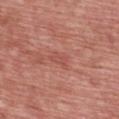The lesion was tiled from a total-body skin photograph and was not biopsied. Imaged with white-light lighting. From the upper back. A roughly 15 mm field-of-view crop from a total-body skin photograph. An algorithmic analysis of the crop reported a lesion–skin lightness drop of about 6 and a normalized border contrast of about 5. And it measured border irregularity of about 4.5 on a 0–10 scale, internal color variation of about 0 on a 0–10 scale, and peripheral color asymmetry of about 0. A male patient, about 60 years old. The recorded lesion diameter is about 2.5 mm.